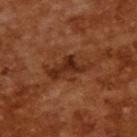Acquisition and patient details:
A male patient, in their mid- to late 60s. Captured under cross-polarized illumination. About 5.5 mm across. An algorithmic analysis of the crop reported a footprint of about 10 mm², an eccentricity of roughly 0.85, and two-axis asymmetry of about 0.35. The software also gave border irregularity of about 5 on a 0–10 scale, a within-lesion color-variation index near 5/10, and a peripheral color-asymmetry measure near 2. The analysis additionally found a nevus-likeness score of about 25/100 and a lesion-detection confidence of about 100/100. A close-up tile cropped from a whole-body skin photograph, about 15 mm across.